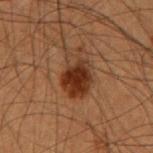| field | value |
|---|---|
| follow-up | catalogued during a skin exam; not biopsied |
| illumination | cross-polarized |
| image | ~15 mm tile from a whole-body skin photo |
| subject | male, aged 53 to 57 |
| automated metrics | an automated nevus-likeness rating near 95 out of 100 |
| lesion size | ~4.5 mm (longest diameter) |
| anatomic site | the right forearm |